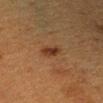| feature | finding |
|---|---|
| biopsy status | imaged on a skin check; not biopsied |
| anatomic site | the right forearm |
| lesion diameter | ~2 mm (longest diameter) |
| automated metrics | a within-lesion color-variation index near 2.5/10 and a peripheral color-asymmetry measure near 1 |
| imaging modality | total-body-photography crop, ~15 mm field of view |
| subject | female, aged 38 to 42 |